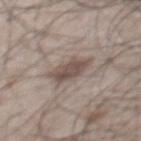Assessment: Imaged during a routine full-body skin examination; the lesion was not biopsied and no histopathology is available. Context: Longest diameter approximately 4 mm. This is a white-light tile. Located on the mid back. A male subject, about 50 years old. A lesion tile, about 15 mm wide, cut from a 3D total-body photograph.